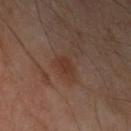<record>
<biopsy_status>not biopsied; imaged during a skin examination</biopsy_status>
<image>
  <source>total-body photography crop</source>
  <field_of_view_mm>15</field_of_view_mm>
</image>
<automated_metrics>
  <vs_skin_darker_L>6.0</vs_skin_darker_L>
  <vs_skin_contrast_norm>6.0</vs_skin_contrast_norm>
</automated_metrics>
<lesion_size>
  <long_diameter_mm_approx>2.5</long_diameter_mm_approx>
</lesion_size>
<lighting>cross-polarized</lighting>
<site>left upper arm</site>
<patient>
  <sex>male</sex>
  <age_approx>55</age_approx>
</patient>
</record>